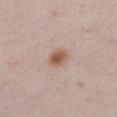The subject is a female approximately 20 years of age. Captured under white-light illumination. A roughly 15 mm field-of-view crop from a total-body skin photograph. From the left lower leg.